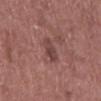| key | value |
|---|---|
| subject | male, aged approximately 60 |
| illumination | white-light |
| image source | ~15 mm crop, total-body skin-cancer survey |
| site | the back |
| diameter | about 3.5 mm |